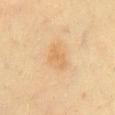biopsy_status: not biopsied; imaged during a skin examination
lesion_size:
  long_diameter_mm_approx: 3.5
patient:
  sex: female
  age_approx: 40
image:
  source: total-body photography crop
  field_of_view_mm: 15
site: lower back